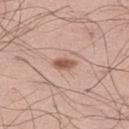The lesion was photographed on a routine skin check and not biopsied; there is no pathology result.
A male subject in their 40s.
On the left thigh.
A 15 mm crop from a total-body photograph taken for skin-cancer surveillance.
This is a white-light tile.
The lesion-visualizer software estimated a lesion–skin lightness drop of about 13 and a lesion-to-skin contrast of about 8.5 (normalized; higher = more distinct). The analysis additionally found a border-irregularity rating of about 2/10, internal color variation of about 1.5 on a 0–10 scale, and a peripheral color-asymmetry measure near 0.5.
About 2.5 mm across.